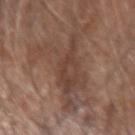This lesion was catalogued during total-body skin photography and was not selected for biopsy.
A male patient, roughly 70 years of age.
The lesion is on the right forearm.
A 15 mm close-up tile from a total-body photography series done for melanoma screening.